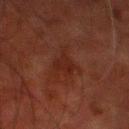Assessment: Captured during whole-body skin photography for melanoma surveillance; the lesion was not biopsied. Clinical summary: Imaged with cross-polarized lighting. Located on the left lower leg. About 2.5 mm across. A 15 mm close-up tile from a total-body photography series done for melanoma screening. A male subject, aged approximately 80.